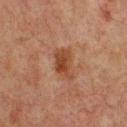Imaged during a routine full-body skin examination; the lesion was not biopsied and no histopathology is available. The lesion is on the chest. Cropped from a total-body skin-imaging series; the visible field is about 15 mm. A male subject aged approximately 60.